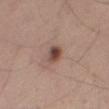follow-up: catalogued during a skin exam; not biopsied
location: the leg
image-analysis metrics: roughly 13 lightness units darker than nearby skin and a normalized border contrast of about 9.5; a border-irregularity rating of about 1.5/10, a within-lesion color-variation index near 5.5/10, and peripheral color asymmetry of about 1.5; lesion-presence confidence of about 100/100
acquisition: ~15 mm crop, total-body skin-cancer survey
lighting: white-light
subject: male, aged 58–62